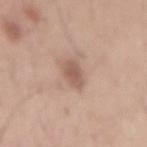Impression:
This lesion was catalogued during total-body skin photography and was not selected for biopsy.
Image and clinical context:
Cropped from a whole-body photographic skin survey; the tile spans about 15 mm. A male patient in their mid- to late 50s. On the mid back. Approximately 3.5 mm at its widest. Captured under white-light illumination.